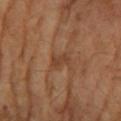workup — imaged on a skin check; not biopsied
diameter — about 2.5 mm
subject — female, approximately 70 years of age
automated metrics — lesion-presence confidence of about 100/100
tile lighting — cross-polarized illumination
acquisition — ~15 mm tile from a whole-body skin photo
location — the left forearm